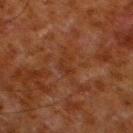Impression:
The lesion was photographed on a routine skin check and not biopsied; there is no pathology result.
Acquisition and patient details:
The lesion is located on the left lower leg. A male subject aged 78 to 82. A lesion tile, about 15 mm wide, cut from a 3D total-body photograph.